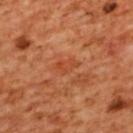Imaged during a routine full-body skin examination; the lesion was not biopsied and no histopathology is available.
This is a cross-polarized tile.
Cropped from a whole-body photographic skin survey; the tile spans about 15 mm.
From the upper back.
The subject is a female about 55 years old.
An algorithmic analysis of the crop reported a lesion-to-skin contrast of about 5 (normalized; higher = more distinct). The software also gave a border-irregularity rating of about 2.5/10 and a peripheral color-asymmetry measure near 0.5.
Approximately 3 mm at its widest.